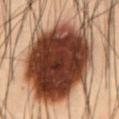biopsy status: catalogued during a skin exam; not biopsied | imaging modality: ~15 mm tile from a whole-body skin photo | tile lighting: cross-polarized | subject: male, aged around 55 | site: the abdomen | lesion size: ~11.5 mm (longest diameter).